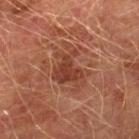biopsy status: catalogued during a skin exam; not biopsied
image source: total-body-photography crop, ~15 mm field of view
lesion size: ~5 mm (longest diameter)
image-analysis metrics: a footprint of about 12 mm² and an eccentricity of roughly 0.7; an automated nevus-likeness rating near 0 out of 100
subject: male, approximately 75 years of age
site: the right lower leg
tile lighting: cross-polarized